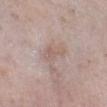Cropped from a whole-body photographic skin survey; the tile spans about 15 mm.
Longest diameter approximately 3.5 mm.
The subject is a male roughly 60 years of age.
Automated tile analysis of the lesion measured a footprint of about 5.5 mm² and an eccentricity of roughly 0.85. It also reported border irregularity of about 4 on a 0–10 scale, internal color variation of about 1.5 on a 0–10 scale, and a peripheral color-asymmetry measure near 0.5. And it measured an automated nevus-likeness rating near 0 out of 100 and a detector confidence of about 90 out of 100 that the crop contains a lesion.
The lesion is on the right lower leg.
Captured under white-light illumination.